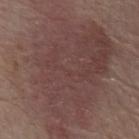This lesion was catalogued during total-body skin photography and was not selected for biopsy. A male subject roughly 80 years of age. The lesion is located on the abdomen. A lesion tile, about 15 mm wide, cut from a 3D total-body photograph. Imaged with white-light lighting. About 16 mm across.